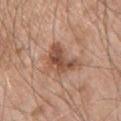Recorded during total-body skin imaging; not selected for excision or biopsy.
An algorithmic analysis of the crop reported an area of roughly 10 mm², an outline eccentricity of about 0.8 (0 = round, 1 = elongated), and two-axis asymmetry of about 0.45. And it measured a lesion color around L≈50 a*≈22 b*≈30 in CIELAB, roughly 12 lightness units darker than nearby skin, and a normalized border contrast of about 8.5. The analysis additionally found a lesion-detection confidence of about 100/100.
The lesion is located on the chest.
A male patient, in their mid- to late 50s.
Cropped from a total-body skin-imaging series; the visible field is about 15 mm.
The lesion's longest dimension is about 5 mm.